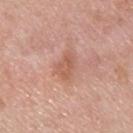biopsy status — imaged on a skin check; not biopsied
size — ≈3 mm
location — the upper back
subject — male, about 35 years old
acquisition — total-body-photography crop, ~15 mm field of view
image-analysis metrics — an average lesion color of about L≈58 a*≈24 b*≈30 (CIELAB) and about 8 CIELAB-L* units darker than the surrounding skin; a classifier nevus-likeness of about 0/100
lighting — white-light